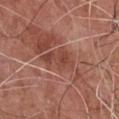No biopsy was performed on this lesion — it was imaged during a full skin examination and was not determined to be concerning.
Longest diameter approximately 4.5 mm.
Captured under white-light illumination.
The lesion is located on the chest.
The patient is a male aged 73–77.
A close-up tile cropped from a whole-body skin photograph, about 15 mm across.